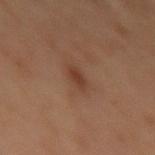The lesion was tiled from a total-body skin photograph and was not biopsied.